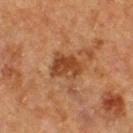Impression:
Part of a total-body skin-imaging series; this lesion was reviewed on a skin check and was not flagged for biopsy.
Image and clinical context:
The lesion's longest dimension is about 4 mm. Cropped from a total-body skin-imaging series; the visible field is about 15 mm. The tile uses cross-polarized illumination. The lesion is located on the upper back. A male subject roughly 50 years of age.